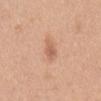No biopsy was performed on this lesion — it was imaged during a full skin examination and was not determined to be concerning.
The lesion-visualizer software estimated a shape eccentricity near 0.9 and a symmetry-axis asymmetry near 0.25. It also reported an average lesion color of about L≈61 a*≈22 b*≈33 (CIELAB), a lesion–skin lightness drop of about 9, and a lesion-to-skin contrast of about 6 (normalized; higher = more distinct). The analysis additionally found a border-irregularity index near 3.5/10. And it measured a lesion-detection confidence of about 100/100.
On the front of the torso.
A female subject, roughly 40 years of age.
This is a white-light tile.
About 3.5 mm across.
A close-up tile cropped from a whole-body skin photograph, about 15 mm across.